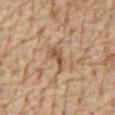Image and clinical context: A male subject, aged 68 to 72. The lesion is located on the front of the torso. About 3 mm across. A 15 mm close-up tile from a total-body photography series done for melanoma screening.